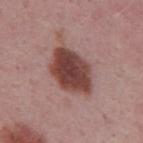Part of a total-body skin-imaging series; this lesion was reviewed on a skin check and was not flagged for biopsy. On the mid back. A male patient, about 50 years old. Imaged with white-light lighting. Measured at roughly 6 mm in maximum diameter. A roughly 15 mm field-of-view crop from a total-body skin photograph.